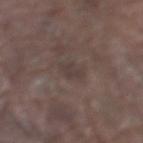Q: Where on the body is the lesion?
A: the left lower leg
Q: What did automated image analysis measure?
A: an average lesion color of about L≈38 a*≈11 b*≈17 (CIELAB), a lesion–skin lightness drop of about 6, and a normalized border contrast of about 5.5; a border-irregularity rating of about 2.5/10 and internal color variation of about 1.5 on a 0–10 scale
Q: What lighting was used for the tile?
A: white-light illumination
Q: What is the imaging modality?
A: ~15 mm tile from a whole-body skin photo
Q: Who is the patient?
A: male, aged around 80
Q: How large is the lesion?
A: ~2.5 mm (longest diameter)